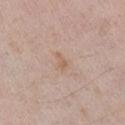Captured during whole-body skin photography for melanoma surveillance; the lesion was not biopsied.
This is a white-light tile.
A male patient in their mid-50s.
The lesion is located on the right upper arm.
A close-up tile cropped from a whole-body skin photograph, about 15 mm across.
The lesion's longest dimension is about 2.5 mm.